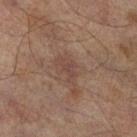{"site": "right lower leg", "lighting": "cross-polarized", "lesion_size": {"long_diameter_mm_approx": 2.5}, "patient": {"sex": "male", "age_approx": 65}, "image": {"source": "total-body photography crop", "field_of_view_mm": 15}}